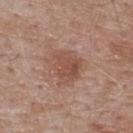Part of a total-body skin-imaging series; this lesion was reviewed on a skin check and was not flagged for biopsy. Located on the upper back. A region of skin cropped from a whole-body photographic capture, roughly 15 mm wide. A male subject, aged 53 to 57.